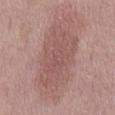workup: catalogued during a skin exam; not biopsied | image source: ~15 mm tile from a whole-body skin photo | tile lighting: white-light | site: the mid back | diameter: ~9.5 mm (longest diameter) | automated metrics: border irregularity of about 4.5 on a 0–10 scale and internal color variation of about 2.5 on a 0–10 scale | subject: male, aged around 55.